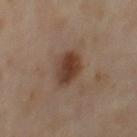Captured during whole-body skin photography for melanoma surveillance; the lesion was not biopsied. Approximately 4 mm at its widest. From the right thigh. Cropped from a total-body skin-imaging series; the visible field is about 15 mm. A female subject in their 50s.